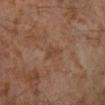Part of a total-body skin-imaging series; this lesion was reviewed on a skin check and was not flagged for biopsy. Approximately 2.5 mm at its widest. A 15 mm crop from a total-body photograph taken for skin-cancer surveillance. A male patient, aged 58 to 62. Imaged with cross-polarized lighting. Located on the left lower leg.